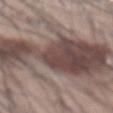{"biopsy_status": "not biopsied; imaged during a skin examination", "image": {"source": "total-body photography crop", "field_of_view_mm": 15}, "patient": {"sex": "male", "age_approx": 45}, "site": "abdomen", "lesion_size": {"long_diameter_mm_approx": 12.0}, "lighting": "white-light"}